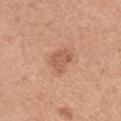Part of a total-body skin-imaging series; this lesion was reviewed on a skin check and was not flagged for biopsy. The subject is a female about 65 years old. A close-up tile cropped from a whole-body skin photograph, about 15 mm across. From the left upper arm. Measured at roughly 3 mm in maximum diameter.